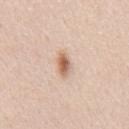The lesion was photographed on a routine skin check and not biopsied; there is no pathology result.